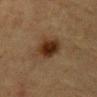Findings:
* follow-up — catalogued during a skin exam; not biopsied
* body site — the front of the torso
* patient — female, in their mid-50s
* illumination — cross-polarized illumination
* imaging modality — 15 mm crop, total-body photography
* lesion diameter — ≈4 mm